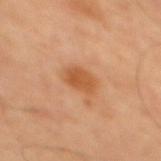Part of a total-body skin-imaging series; this lesion was reviewed on a skin check and was not flagged for biopsy.
A male subject approximately 65 years of age.
Located on the mid back.
A roughly 15 mm field-of-view crop from a total-body skin photograph.